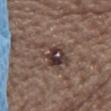Context:
Located on the upper back. Automated image analysis of the tile measured a lesion area of about 10 mm², an eccentricity of roughly 0.75, and a symmetry-axis asymmetry near 0.45. The analysis additionally found an average lesion color of about L≈38 a*≈14 b*≈19 (CIELAB), roughly 10 lightness units darker than nearby skin, and a lesion-to-skin contrast of about 9 (normalized; higher = more distinct). A close-up tile cropped from a whole-body skin photograph, about 15 mm across. This is a white-light tile. A female subject, aged 63–67.